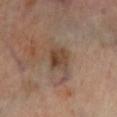<lesion>
  <biopsy_status>not biopsied; imaged during a skin examination</biopsy_status>
  <image>
    <source>total-body photography crop</source>
    <field_of_view_mm>15</field_of_view_mm>
  </image>
  <lighting>cross-polarized</lighting>
  <patient>
    <sex>male</sex>
    <age_approx>70</age_approx>
  </patient>
  <lesion_size>
    <long_diameter_mm_approx>3.0</long_diameter_mm_approx>
  </lesion_size>
  <automated_metrics>
    <border_irregularity_0_10>2.0</border_irregularity_0_10>
    <color_variation_0_10>5.5</color_variation_0_10>
    <peripheral_color_asymmetry>2.0</peripheral_color_asymmetry>
  </automated_metrics>
  <site>left lower leg</site>
</lesion>